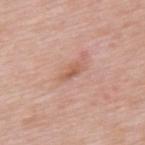biopsy status = no biopsy performed (imaged during a skin exam)
diameter = ≈2.5 mm
tile lighting = white-light
subject = male, in their mid- to late 50s
automated lesion analysis = a lesion–skin lightness drop of about 9 and a normalized lesion–skin contrast near 6.5; a color-variation rating of about 1/10 and a peripheral color-asymmetry measure near 0
body site = the mid back
imaging modality = ~15 mm tile from a whole-body skin photo